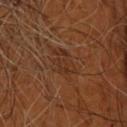workup: no biopsy performed (imaged during a skin exam)
location: the head or neck
patient: male, in their mid- to late 50s
diameter: ≈5 mm
image source: ~15 mm tile from a whole-body skin photo
TBP lesion metrics: a nevus-likeness score of about 0/100